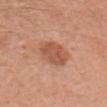Assessment: Imaged during a routine full-body skin examination; the lesion was not biopsied and no histopathology is available. Context: A female subject, aged around 65. The total-body-photography lesion software estimated a footprint of about 11 mm² and a shape-asymmetry score of about 0.1 (0 = symmetric). The software also gave a lesion color around L≈55 a*≈25 b*≈32 in CIELAB and about 10 CIELAB-L* units darker than the surrounding skin. And it measured a border-irregularity rating of about 1/10, a within-lesion color-variation index near 3/10, and peripheral color asymmetry of about 1. The software also gave a classifier nevus-likeness of about 55/100. Cropped from a whole-body photographic skin survey; the tile spans about 15 mm. Located on the head or neck. Measured at roughly 4 mm in maximum diameter. Captured under white-light illumination.